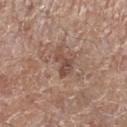biopsy status = no biopsy performed (imaged during a skin exam) | tile lighting = white-light illumination | anatomic site = the right lower leg | subject = male, about 70 years old | lesion size = about 3.5 mm | image = total-body-photography crop, ~15 mm field of view.